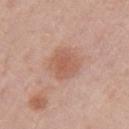  lighting: white-light
  lesion_size:
    long_diameter_mm_approx: 4.0
  image:
    source: total-body photography crop
    field_of_view_mm: 15
  site: arm
  patient:
    sex: male
    age_approx: 70